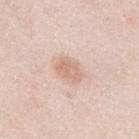An algorithmic analysis of the crop reported an outline eccentricity of about 0.8 (0 = round, 1 = elongated) and two-axis asymmetry of about 0.25. The analysis additionally found an average lesion color of about L≈69 a*≈19 b*≈28 (CIELAB), about 9 CIELAB-L* units darker than the surrounding skin, and a normalized lesion–skin contrast near 6. A close-up tile cropped from a whole-body skin photograph, about 15 mm across. On the mid back. A female subject in their 40s.